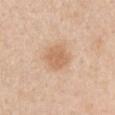{
  "biopsy_status": "not biopsied; imaged during a skin examination",
  "site": "left upper arm",
  "lighting": "white-light",
  "image": {
    "source": "total-body photography crop",
    "field_of_view_mm": 15
  },
  "lesion_size": {
    "long_diameter_mm_approx": 3.0
  },
  "automated_metrics": {
    "area_mm2_approx": 7.5,
    "eccentricity": 0.4,
    "shape_asymmetry": 0.15,
    "cielab_L": 64,
    "cielab_a": 20,
    "cielab_b": 34,
    "vs_skin_contrast_norm": 6.5,
    "lesion_detection_confidence_0_100": 100
  },
  "patient": {
    "sex": "male",
    "age_approx": 60
  }
}